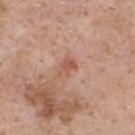Notes:
- follow-up: no biopsy performed (imaged during a skin exam)
- lesion size: ~2.5 mm (longest diameter)
- lighting: white-light illumination
- location: the upper back
- patient: male, roughly 60 years of age
- automated lesion analysis: a footprint of about 3.5 mm², a shape eccentricity near 0.75, and two-axis asymmetry of about 0.35; a mean CIELAB color near L≈55 a*≈24 b*≈29, roughly 8 lightness units darker than nearby skin, and a lesion-to-skin contrast of about 6 (normalized; higher = more distinct)
- acquisition: ~15 mm crop, total-body skin-cancer survey